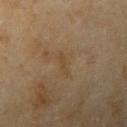This lesion was catalogued during total-body skin photography and was not selected for biopsy. The recorded lesion diameter is about 3 mm. A 15 mm close-up extracted from a 3D total-body photography capture. A female patient aged 58 to 62. An algorithmic analysis of the crop reported a lesion color around L≈40 a*≈13 b*≈31 in CIELAB. It also reported a color-variation rating of about 0/10 and peripheral color asymmetry of about 0. The software also gave a classifier nevus-likeness of about 0/100 and a lesion-detection confidence of about 100/100. From the right upper arm. Captured under cross-polarized illumination.